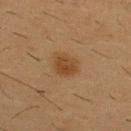Recorded during total-body skin imaging; not selected for excision or biopsy.
The patient is a male in their mid- to late 50s.
Located on the chest.
This image is a 15 mm lesion crop taken from a total-body photograph.